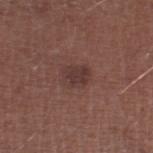Imaged during a routine full-body skin examination; the lesion was not biopsied and no histopathology is available. A close-up tile cropped from a whole-body skin photograph, about 15 mm across. On the right lower leg. The lesion's longest dimension is about 3 mm. The subject is a male in their mid-60s. The lesion-visualizer software estimated a mean CIELAB color near L≈36 a*≈19 b*≈20, roughly 7 lightness units darker than nearby skin, and a normalized lesion–skin contrast near 6.5. The software also gave border irregularity of about 2 on a 0–10 scale and a peripheral color-asymmetry measure near 0.5. It also reported a classifier nevus-likeness of about 20/100.